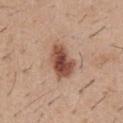• follow-up: imaged on a skin check; not biopsied
• imaging modality: ~15 mm crop, total-body skin-cancer survey
• site: the front of the torso
• subject: male, about 30 years old